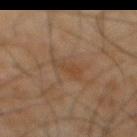Imaged during a routine full-body skin examination; the lesion was not biopsied and no histopathology is available. Located on the front of the torso. The total-body-photography lesion software estimated an area of roughly 3.5 mm², an outline eccentricity of about 0.9 (0 = round, 1 = elongated), and a symmetry-axis asymmetry near 0.45. It also reported a lesion–skin lightness drop of about 5 and a normalized border contrast of about 5.5. The software also gave a within-lesion color-variation index near 1/10 and peripheral color asymmetry of about 0. The software also gave a nevus-likeness score of about 0/100. Cropped from a whole-body photographic skin survey; the tile spans about 15 mm. Imaged with cross-polarized lighting. A male patient, roughly 65 years of age. The recorded lesion diameter is about 3.5 mm.